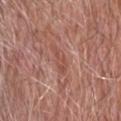Q: Is there a histopathology result?
A: catalogued during a skin exam; not biopsied
Q: Patient demographics?
A: male, approximately 80 years of age
Q: What kind of image is this?
A: 15 mm crop, total-body photography
Q: Automated lesion metrics?
A: a shape eccentricity near 0.9 and a symmetry-axis asymmetry near 0.35; border irregularity of about 4 on a 0–10 scale, internal color variation of about 2 on a 0–10 scale, and a peripheral color-asymmetry measure near 0.5
Q: How large is the lesion?
A: ~3.5 mm (longest diameter)
Q: How was the tile lit?
A: white-light illumination
Q: Lesion location?
A: the head or neck